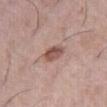Clinical impression:
Captured during whole-body skin photography for melanoma surveillance; the lesion was not biopsied.
Context:
This is a white-light tile. The total-body-photography lesion software estimated a mean CIELAB color near L≈52 a*≈20 b*≈24, about 12 CIELAB-L* units darker than the surrounding skin, and a normalized lesion–skin contrast near 8.5. And it measured an automated nevus-likeness rating near 50 out of 100 and lesion-presence confidence of about 100/100. Measured at roughly 2.5 mm in maximum diameter. The subject is a female roughly 60 years of age. From the right thigh. A close-up tile cropped from a whole-body skin photograph, about 15 mm across.